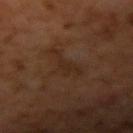Image and clinical context: Measured at roughly 5 mm in maximum diameter. The lesion is on the right upper arm. A roughly 15 mm field-of-view crop from a total-body skin photograph. A male patient, roughly 60 years of age. This is a cross-polarized tile.